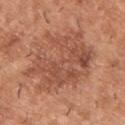The lesion was tiled from a total-body skin photograph and was not biopsied. The lesion-visualizer software estimated a footprint of about 36 mm² and an outline eccentricity of about 0.55 (0 = round, 1 = elongated). It also reported a lesion–skin lightness drop of about 9 and a lesion-to-skin contrast of about 6.5 (normalized; higher = more distinct). The software also gave border irregularity of about 7 on a 0–10 scale, a color-variation rating of about 5/10, and a peripheral color-asymmetry measure near 2. The software also gave a nevus-likeness score of about 45/100 and lesion-presence confidence of about 100/100. Approximately 9 mm at its widest. A close-up tile cropped from a whole-body skin photograph, about 15 mm across. This is a white-light tile. The patient is a male approximately 40 years of age. From the upper back.